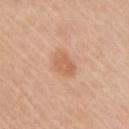  biopsy_status: not biopsied; imaged during a skin examination
  patient:
    sex: female
    age_approx: 45
  automated_metrics:
    area_mm2_approx: 5.0
    eccentricity: 0.45
    cielab_L: 61
    cielab_a: 24
    cielab_b: 35
    vs_skin_contrast_norm: 6.0
    border_irregularity_0_10: 2.5
    color_variation_0_10: 2.0
    nevus_likeness_0_100: 70
    lesion_detection_confidence_0_100: 100
  site: right upper arm
  image:
    source: total-body photography crop
    field_of_view_mm: 15
  lighting: white-light
  lesion_size:
    long_diameter_mm_approx: 2.5